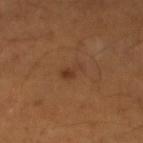Recorded during total-body skin imaging; not selected for excision or biopsy. Longest diameter approximately 3 mm. On the left lower leg. Captured under cross-polarized illumination. A 15 mm close-up tile from a total-body photography series done for melanoma screening. The subject is a male aged 53–57.